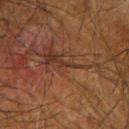Assessment: Imaged during a routine full-body skin examination; the lesion was not biopsied and no histopathology is available. Background: A male patient, in their mid- to late 60s. On the right forearm. This is a cross-polarized tile. Cropped from a whole-body photographic skin survey; the tile spans about 15 mm. About 1 mm across. An algorithmic analysis of the crop reported a footprint of about 0.5 mm², a shape eccentricity near 0.85, and a shape-asymmetry score of about 0.5 (0 = symmetric). It also reported a mean CIELAB color near L≈25 a*≈16 b*≈24 and roughly 6 lightness units darker than nearby skin. The analysis additionally found a classifier nevus-likeness of about 0/100 and a detector confidence of about 0 out of 100 that the crop contains a lesion.